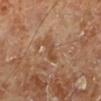Clinical impression: This lesion was catalogued during total-body skin photography and was not selected for biopsy. Context: Located on the left lower leg. A male subject, roughly 70 years of age. A region of skin cropped from a whole-body photographic capture, roughly 15 mm wide.